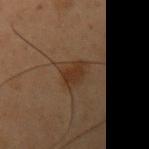The lesion was tiled from a total-body skin photograph and was not biopsied.
Cropped from a whole-body photographic skin survey; the tile spans about 15 mm.
A male patient, in their mid-50s.
Approximately 3.5 mm at its widest.
From the left upper arm.
The tile uses cross-polarized illumination.